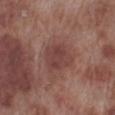No biopsy was performed on this lesion — it was imaged during a full skin examination and was not determined to be concerning. The lesion is located on the leg. Approximately 4 mm at its widest. The patient is a male in their 70s. A 15 mm crop from a total-body photograph taken for skin-cancer surveillance. Imaged with white-light lighting.